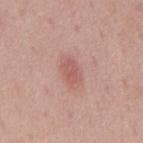A male patient, in their mid-40s.
The lesion is on the mid back.
This image is a 15 mm lesion crop taken from a total-body photograph.
The recorded lesion diameter is about 3 mm.
Imaged with white-light lighting.
The total-body-photography lesion software estimated an automated nevus-likeness rating near 65 out of 100.